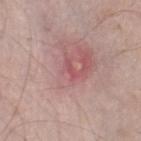Recorded during total-body skin imaging; not selected for excision or biopsy. The lesion is on the right lower leg. Captured under white-light illumination. The patient is a male aged 58–62. A roughly 15 mm field-of-view crop from a total-body skin photograph.